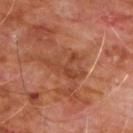| feature | finding |
|---|---|
| biopsy status | imaged on a skin check; not biopsied |
| image-analysis metrics | a lesion area of about 8.5 mm² and a shape eccentricity near 0.8; a within-lesion color-variation index near 4.5/10 and a peripheral color-asymmetry measure near 1.5; a lesion-detection confidence of about 75/100 |
| size | ~5.5 mm (longest diameter) |
| lighting | cross-polarized illumination |
| acquisition | ~15 mm tile from a whole-body skin photo |
| subject | male, aged 58–62 |
| site | the chest |